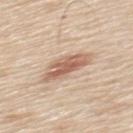Imaged during a routine full-body skin examination; the lesion was not biopsied and no histopathology is available.
On the mid back.
The lesion-visualizer software estimated an average lesion color of about L≈61 a*≈19 b*≈30 (CIELAB) and roughly 12 lightness units darker than nearby skin. The software also gave border irregularity of about 3.5 on a 0–10 scale.
About 5 mm across.
Imaged with white-light lighting.
A close-up tile cropped from a whole-body skin photograph, about 15 mm across.
A male subject, approximately 60 years of age.